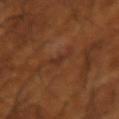<lesion>
<lesion_size>
  <long_diameter_mm_approx>2.5</long_diameter_mm_approx>
</lesion_size>
<site>right forearm</site>
<lighting>cross-polarized</lighting>
<image>
  <source>total-body photography crop</source>
  <field_of_view_mm>15</field_of_view_mm>
</image>
<patient>
  <sex>male</sex>
  <age_approx>65</age_approx>
</patient>
<automated_metrics>
  <cielab_L>31</cielab_L>
  <cielab_a>22</cielab_a>
  <cielab_b>29</cielab_b>
  <vs_skin_darker_L>5.0</vs_skin_darker_L>
  <vs_skin_contrast_norm>6.0</vs_skin_contrast_norm>
  <border_irregularity_0_10>5.0</border_irregularity_0_10>
  <color_variation_0_10>0.0</color_variation_0_10>
  <peripheral_color_asymmetry>0.0</peripheral_color_asymmetry>
  <nevus_likeness_0_100>0</nevus_likeness_0_100>
  <lesion_detection_confidence_0_100>100</lesion_detection_confidence_0_100>
</automated_metrics>
</lesion>